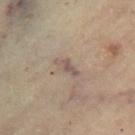workup=catalogued during a skin exam; not biopsied
diameter=≈3 mm
image source=15 mm crop, total-body photography
body site=the right thigh
tile lighting=cross-polarized
subject=female, roughly 60 years of age